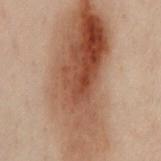Recorded during total-body skin imaging; not selected for excision or biopsy.
Automated tile analysis of the lesion measured an outline eccentricity of about 0.95 (0 = round, 1 = elongated) and two-axis asymmetry of about 0.25. The software also gave an average lesion color of about L≈42 a*≈17 b*≈26 (CIELAB). It also reported an automated nevus-likeness rating near 90 out of 100.
A male subject, aged around 50.
Imaged with cross-polarized lighting.
From the chest.
A region of skin cropped from a whole-body photographic capture, roughly 15 mm wide.
Measured at roughly 17 mm in maximum diameter.